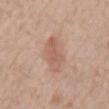The lesion was photographed on a routine skin check and not biopsied; there is no pathology result. Longest diameter approximately 4.5 mm. Cropped from a whole-body photographic skin survey; the tile spans about 15 mm. A male patient aged around 80. The tile uses white-light illumination. On the back.